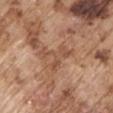Impression: This lesion was catalogued during total-body skin photography and was not selected for biopsy. Acquisition and patient details: The lesion-visualizer software estimated two-axis asymmetry of about 0.8. And it measured an average lesion color of about L≈52 a*≈21 b*≈32 (CIELAB), a lesion–skin lightness drop of about 8, and a normalized lesion–skin contrast near 5.5. Located on the right upper arm. Approximately 4 mm at its widest. A male patient approximately 75 years of age. A roughly 15 mm field-of-view crop from a total-body skin photograph. The tile uses white-light illumination.